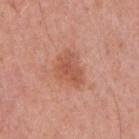The lesion was tiled from a total-body skin photograph and was not biopsied.
The patient is a male in their mid-50s.
A roughly 15 mm field-of-view crop from a total-body skin photograph.
The tile uses white-light illumination.
The lesion's longest dimension is about 4.5 mm.
The total-body-photography lesion software estimated a footprint of about 9.5 mm², a shape eccentricity near 0.7, and a symmetry-axis asymmetry near 0.3. And it measured lesion-presence confidence of about 100/100.
On the right upper arm.